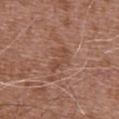notes: no biopsy performed (imaged during a skin exam)
image-analysis metrics: a lesion area of about 4.5 mm², an outline eccentricity of about 0.9 (0 = round, 1 = elongated), and two-axis asymmetry of about 0.3; a mean CIELAB color near L≈47 a*≈21 b*≈29, a lesion–skin lightness drop of about 7, and a normalized lesion–skin contrast near 5.5; a border-irregularity index near 4.5/10, a within-lesion color-variation index near 2/10, and radial color variation of about 0.5; an automated nevus-likeness rating near 0 out of 100 and a lesion-detection confidence of about 100/100
patient: male, aged 73 to 77
location: the abdomen
image source: total-body-photography crop, ~15 mm field of view
tile lighting: white-light